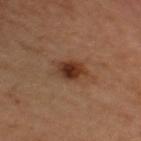notes: catalogued during a skin exam; not biopsied | imaging modality: 15 mm crop, total-body photography | size: about 3.5 mm | body site: the right thigh | patient: male, in their mid-60s | lighting: cross-polarized | TBP lesion metrics: a mean CIELAB color near L≈35 a*≈21 b*≈29, about 11 CIELAB-L* units darker than the surrounding skin, and a normalized border contrast of about 10; an automated nevus-likeness rating near 95 out of 100.